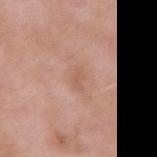<record>
  <biopsy_status>not biopsied; imaged during a skin examination</biopsy_status>
  <lesion_size>
    <long_diameter_mm_approx>2.5</long_diameter_mm_approx>
  </lesion_size>
  <lighting>white-light</lighting>
  <site>right upper arm</site>
  <patient>
    <sex>male</sex>
    <age_approx>55</age_approx>
  </patient>
  <automated_metrics>
    <area_mm2_approx>3.5</area_mm2_approx>
    <eccentricity>0.8</eccentricity>
    <shape_asymmetry>0.25</shape_asymmetry>
    <border_irregularity_0_10>3.0</border_irregularity_0_10>
    <color_variation_0_10>1.0</color_variation_0_10>
    <peripheral_color_asymmetry>0.5</peripheral_color_asymmetry>
  </automated_metrics>
  <image>
    <source>total-body photography crop</source>
    <field_of_view_mm>15</field_of_view_mm>
  </image>
</record>